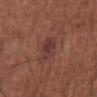Impression:
No biopsy was performed on this lesion — it was imaged during a full skin examination and was not determined to be concerning.
Background:
Measured at roughly 3.5 mm in maximum diameter. Cropped from a total-body skin-imaging series; the visible field is about 15 mm. Captured under white-light illumination. A male subject approximately 65 years of age. The lesion-visualizer software estimated an eccentricity of roughly 0.6 and a symmetry-axis asymmetry near 0.2. The software also gave a nevus-likeness score of about 30/100. The lesion is located on the abdomen.